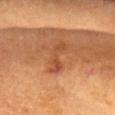{
  "biopsy_status": "not biopsied; imaged during a skin examination",
  "lesion_size": {
    "long_diameter_mm_approx": 4.5
  },
  "image": {
    "source": "total-body photography crop",
    "field_of_view_mm": 15
  },
  "patient": {
    "sex": "male",
    "age_approx": "85+"
  },
  "site": "head or neck",
  "lighting": "cross-polarized",
  "automated_metrics": {
    "vs_skin_darker_L": 7.0
  }
}